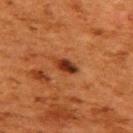Recorded during total-body skin imaging; not selected for excision or biopsy.